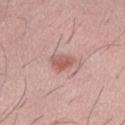No biopsy was performed on this lesion — it was imaged during a full skin examination and was not determined to be concerning. Measured at roughly 3 mm in maximum diameter. From the right thigh. An algorithmic analysis of the crop reported a footprint of about 5 mm² and an eccentricity of roughly 0.75. It also reported a color-variation rating of about 3/10 and a peripheral color-asymmetry measure near 1. A roughly 15 mm field-of-view crop from a total-body skin photograph. A male patient, about 40 years old. Captured under white-light illumination.